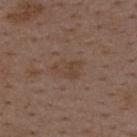This is a white-light tile.
A male subject, approximately 50 years of age.
A region of skin cropped from a whole-body photographic capture, roughly 15 mm wide.
From the mid back.